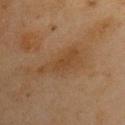Findings:
- workup: catalogued during a skin exam; not biopsied
- patient: male, in their mid- to late 40s
- lesion diameter: ≈5.5 mm
- site: the chest
- image: ~15 mm tile from a whole-body skin photo
- illumination: cross-polarized
- image-analysis metrics: an outline eccentricity of about 0.95 (0 = round, 1 = elongated) and two-axis asymmetry of about 0.4; border irregularity of about 5.5 on a 0–10 scale and peripheral color asymmetry of about 0.5